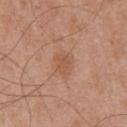follow-up = catalogued during a skin exam; not biopsied
size = ≈3 mm
patient = male, in their mid- to late 70s
acquisition = 15 mm crop, total-body photography
lighting = white-light illumination
anatomic site = the front of the torso